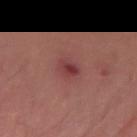Impression: Recorded during total-body skin imaging; not selected for excision or biopsy. Context: A male patient in their mid-60s. A 15 mm close-up tile from a total-body photography series done for melanoma screening. The lesion is located on the right forearm.